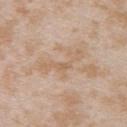{
  "biopsy_status": "not biopsied; imaged during a skin examination",
  "lesion_size": {
    "long_diameter_mm_approx": 6.0
  },
  "patient": {
    "sex": "female",
    "age_approx": 25
  },
  "image": {
    "source": "total-body photography crop",
    "field_of_view_mm": 15
  },
  "site": "upper back",
  "lighting": "white-light"
}